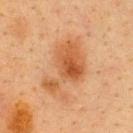Clinical impression:
Recorded during total-body skin imaging; not selected for excision or biopsy.
Clinical summary:
The patient is a female about 40 years old. On the upper back. A close-up tile cropped from a whole-body skin photograph, about 15 mm across. About 7 mm across. The tile uses cross-polarized illumination.